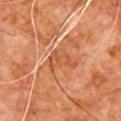This lesion was catalogued during total-body skin photography and was not selected for biopsy. The lesion is on the chest. Captured under cross-polarized illumination. The lesion-visualizer software estimated a border-irregularity index near 5/10, a within-lesion color-variation index near 2/10, and a peripheral color-asymmetry measure near 0.5. It also reported a nevus-likeness score of about 0/100 and lesion-presence confidence of about 95/100. A male patient aged around 80. Cropped from a total-body skin-imaging series; the visible field is about 15 mm.